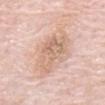Case summary:
• follow-up: total-body-photography surveillance lesion; no biopsy
• subject: male, aged 78 to 82
• location: the front of the torso
• acquisition: ~15 mm tile from a whole-body skin photo
• lighting: white-light illumination
• size: ≈5.5 mm